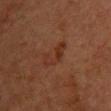Q: Was this lesion biopsied?
A: catalogued during a skin exam; not biopsied
Q: What are the patient's age and sex?
A: female, aged around 50
Q: What kind of image is this?
A: ~15 mm tile from a whole-body skin photo
Q: Where on the body is the lesion?
A: the chest
Q: How was the tile lit?
A: cross-polarized illumination
Q: Lesion size?
A: about 4 mm